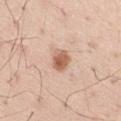Part of a total-body skin-imaging series; this lesion was reviewed on a skin check and was not flagged for biopsy.
The lesion is located on the abdomen.
A close-up tile cropped from a whole-body skin photograph, about 15 mm across.
The tile uses white-light illumination.
A male patient, aged 53 to 57.